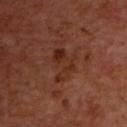biopsy status: total-body-photography surveillance lesion; no biopsy | patient: male, aged 48 to 52 | body site: the back | imaging modality: ~15 mm crop, total-body skin-cancer survey | image-analysis metrics: a color-variation rating of about 3.5/10 and peripheral color asymmetry of about 1 | tile lighting: cross-polarized illumination.